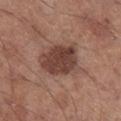biopsy status = imaged on a skin check; not biopsied | location = the right lower leg | patient = male, aged 53–57 | image source = total-body-photography crop, ~15 mm field of view.